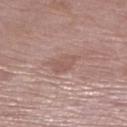Notes:
- notes — catalogued during a skin exam; not biopsied
- image source — total-body-photography crop, ~15 mm field of view
- size — about 3 mm
- patient — female, roughly 70 years of age
- site — the right lower leg